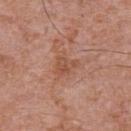Impression:
This lesion was catalogued during total-body skin photography and was not selected for biopsy.
Acquisition and patient details:
Captured under white-light illumination. An algorithmic analysis of the crop reported an area of roughly 5 mm², an eccentricity of roughly 0.6, and two-axis asymmetry of about 0.4. It also reported an average lesion color of about L≈51 a*≈23 b*≈30 (CIELAB) and a lesion–skin lightness drop of about 7. It also reported border irregularity of about 3.5 on a 0–10 scale, internal color variation of about 3.5 on a 0–10 scale, and peripheral color asymmetry of about 1.5. A close-up tile cropped from a whole-body skin photograph, about 15 mm across. A male subject aged around 70. The lesion is on the chest. The lesion's longest dimension is about 3 mm.